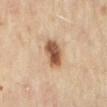| feature | finding |
|---|---|
| follow-up | no biopsy performed (imaged during a skin exam) |
| patient | female, aged around 60 |
| tile lighting | cross-polarized |
| lesion diameter | ≈4 mm |
| location | the back |
| image | ~15 mm crop, total-body skin-cancer survey |
| TBP lesion metrics | an average lesion color of about L≈55 a*≈19 b*≈33 (CIELAB) and a lesion-to-skin contrast of about 10.5 (normalized; higher = more distinct) |